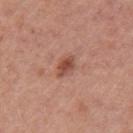Q: Is there a histopathology result?
A: total-body-photography surveillance lesion; no biopsy
Q: Automated lesion metrics?
A: a mean CIELAB color near L≈49 a*≈25 b*≈29, roughly 12 lightness units darker than nearby skin, and a normalized lesion–skin contrast near 8.5
Q: Lesion size?
A: ~2.5 mm (longest diameter)
Q: What lighting was used for the tile?
A: white-light illumination
Q: What is the anatomic site?
A: the left upper arm
Q: How was this image acquired?
A: 15 mm crop, total-body photography
Q: Patient demographics?
A: female, roughly 40 years of age